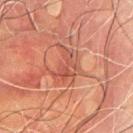The lesion was photographed on a routine skin check and not biopsied; there is no pathology result. A close-up tile cropped from a whole-body skin photograph, about 15 mm across. An algorithmic analysis of the crop reported a lesion area of about 9.5 mm², a shape eccentricity near 0.7, and two-axis asymmetry of about 0.55. The software also gave a border-irregularity rating of about 6/10, a color-variation rating of about 4.5/10, and radial color variation of about 1.5. And it measured a detector confidence of about 95 out of 100 that the crop contains a lesion. Located on the mid back. The subject is a male aged around 70. Captured under cross-polarized illumination. The recorded lesion diameter is about 4.5 mm.